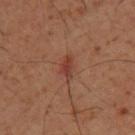Q: Is there a histopathology result?
A: total-body-photography surveillance lesion; no biopsy
Q: What is the imaging modality?
A: ~15 mm crop, total-body skin-cancer survey
Q: Where on the body is the lesion?
A: the upper back
Q: What are the patient's age and sex?
A: male, aged around 50